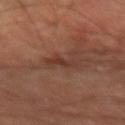Part of a total-body skin-imaging series; this lesion was reviewed on a skin check and was not flagged for biopsy.
A close-up tile cropped from a whole-body skin photograph, about 15 mm across.
From the right forearm.
About 3.5 mm across.
Imaged with cross-polarized lighting.
A male subject aged 58–62.
Automated image analysis of the tile measured an area of roughly 4.5 mm² and an outline eccentricity of about 0.85 (0 = round, 1 = elongated). The analysis additionally found a mean CIELAB color near L≈35 a*≈20 b*≈25 and about 7 CIELAB-L* units darker than the surrounding skin. The software also gave a border-irregularity index near 4.5/10, a within-lesion color-variation index near 3/10, and a peripheral color-asymmetry measure near 1. And it measured a classifier nevus-likeness of about 0/100.